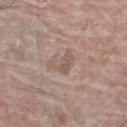Imaged during a routine full-body skin examination; the lesion was not biopsied and no histopathology is available.
Approximately 3 mm at its widest.
The subject is a female approximately 75 years of age.
Automated tile analysis of the lesion measured an area of roughly 4.5 mm² and a symmetry-axis asymmetry near 0.65. And it measured a mean CIELAB color near L≈55 a*≈15 b*≈24, a lesion–skin lightness drop of about 7, and a normalized lesion–skin contrast near 5. The software also gave an automated nevus-likeness rating near 0 out of 100 and a lesion-detection confidence of about 100/100.
Located on the right lower leg.
A 15 mm close-up tile from a total-body photography series done for melanoma screening.